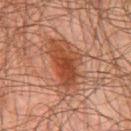notes = imaged on a skin check; not biopsied.